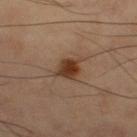Q: Is there a histopathology result?
A: catalogued during a skin exam; not biopsied
Q: What is the anatomic site?
A: the right thigh
Q: What did automated image analysis measure?
A: a shape eccentricity near 0.5 and a symmetry-axis asymmetry near 0.25; internal color variation of about 4.5 on a 0–10 scale and a peripheral color-asymmetry measure near 1.5
Q: Who is the patient?
A: male, aged around 60
Q: How large is the lesion?
A: ≈3 mm
Q: What is the imaging modality?
A: 15 mm crop, total-body photography